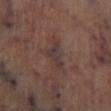Clinical impression: Imaged during a routine full-body skin examination; the lesion was not biopsied and no histopathology is available.